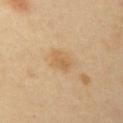Part of a total-body skin-imaging series; this lesion was reviewed on a skin check and was not flagged for biopsy. About 3 mm across. The subject is a female aged approximately 40. A 15 mm close-up extracted from a 3D total-body photography capture. The lesion is located on the left upper arm. The total-body-photography lesion software estimated a border-irregularity rating of about 2.5/10, a within-lesion color-variation index near 2.5/10, and a peripheral color-asymmetry measure near 1. And it measured an automated nevus-likeness rating near 0 out of 100 and lesion-presence confidence of about 100/100.